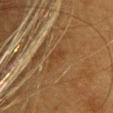Q: Illumination type?
A: cross-polarized
Q: Where on the body is the lesion?
A: the front of the torso
Q: Who is the patient?
A: female, aged around 40
Q: How was this image acquired?
A: ~15 mm crop, total-body skin-cancer survey